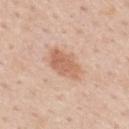Q: Is there a histopathology result?
A: total-body-photography surveillance lesion; no biopsy
Q: What is the lesion's diameter?
A: ~4.5 mm (longest diameter)
Q: What kind of image is this?
A: ~15 mm tile from a whole-body skin photo
Q: What are the patient's age and sex?
A: male, roughly 60 years of age
Q: Where on the body is the lesion?
A: the mid back
Q: How was the tile lit?
A: white-light